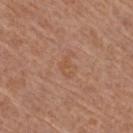Part of a total-body skin-imaging series; this lesion was reviewed on a skin check and was not flagged for biopsy. A female patient, roughly 55 years of age. Automated tile analysis of the lesion measured a lesion area of about 3 mm², an eccentricity of roughly 0.85, and a symmetry-axis asymmetry near 0.6. It also reported a nevus-likeness score of about 0/100 and lesion-presence confidence of about 100/100. A roughly 15 mm field-of-view crop from a total-body skin photograph. On the right thigh. Imaged with white-light lighting. The lesion's longest dimension is about 3 mm.